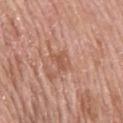<lesion>
<biopsy_status>not biopsied; imaged during a skin examination</biopsy_status>
<lesion_size>
  <long_diameter_mm_approx>2.5</long_diameter_mm_approx>
</lesion_size>
<lighting>white-light</lighting>
<patient>
  <sex>female</sex>
  <age_approx>60</age_approx>
</patient>
<automated_metrics>
  <border_irregularity_0_10>3.5</border_irregularity_0_10>
  <color_variation_0_10>2.0</color_variation_0_10>
  <peripheral_color_asymmetry>0.5</peripheral_color_asymmetry>
  <nevus_likeness_0_100>0</nevus_likeness_0_100>
  <lesion_detection_confidence_0_100>100</lesion_detection_confidence_0_100>
</automated_metrics>
<image>
  <source>total-body photography crop</source>
  <field_of_view_mm>15</field_of_view_mm>
</image>
<site>mid back</site>
</lesion>